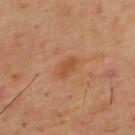Clinical impression: This lesion was catalogued during total-body skin photography and was not selected for biopsy. Background: The lesion is located on the upper back. The subject is a male about 55 years old. An algorithmic analysis of the crop reported a shape eccentricity near 0.85 and two-axis asymmetry of about 0.2. And it measured a lesion color around L≈49 a*≈24 b*≈35 in CIELAB, roughly 7 lightness units darker than nearby skin, and a normalized lesion–skin contrast near 6. A close-up tile cropped from a whole-body skin photograph, about 15 mm across.